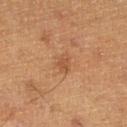The lesion was tiled from a total-body skin photograph and was not biopsied. The tile uses cross-polarized illumination. The lesion is located on the right lower leg. A 15 mm crop from a total-body photograph taken for skin-cancer surveillance. The patient is a male aged 58 to 62. Measured at roughly 2.5 mm in maximum diameter.